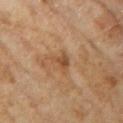The lesion is on the right upper arm.
The patient is a female in their 60s.
A 15 mm crop from a total-body photograph taken for skin-cancer surveillance.